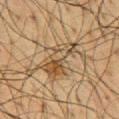notes=total-body-photography surveillance lesion; no biopsy
location=the chest
imaging modality=total-body-photography crop, ~15 mm field of view
patient=male, roughly 60 years of age